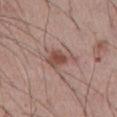Context:
The lesion is located on the abdomen. Longest diameter approximately 4 mm. A 15 mm crop from a total-body photograph taken for skin-cancer surveillance. Imaged with white-light lighting. The subject is a male aged approximately 55. An algorithmic analysis of the crop reported a mean CIELAB color near L≈49 a*≈20 b*≈24, roughly 10 lightness units darker than nearby skin, and a lesion-to-skin contrast of about 8 (normalized; higher = more distinct). The software also gave border irregularity of about 4.5 on a 0–10 scale and a peripheral color-asymmetry measure near 1. The analysis additionally found a lesion-detection confidence of about 100/100.